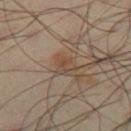{
  "lighting": "cross-polarized",
  "patient": {
    "sex": "male",
    "age_approx": 40
  },
  "automated_metrics": {
    "vs_skin_darker_L": 7.0
  },
  "lesion_size": {
    "long_diameter_mm_approx": 2.5
  },
  "site": "right thigh",
  "image": {
    "source": "total-body photography crop",
    "field_of_view_mm": 15
  }
}